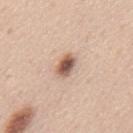Impression: Imaged during a routine full-body skin examination; the lesion was not biopsied and no histopathology is available. Background: A male subject, aged around 45. The tile uses white-light illumination. A 15 mm crop from a total-body photograph taken for skin-cancer surveillance. Approximately 3 mm at its widest. The lesion is on the mid back. Automated image analysis of the tile measured a nevus-likeness score of about 95/100 and a lesion-detection confidence of about 100/100.